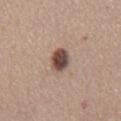Impression:
Recorded during total-body skin imaging; not selected for excision or biopsy.
Clinical summary:
The lesion is on the front of the torso. Captured under white-light illumination. The patient is a male in their mid- to late 50s. A lesion tile, about 15 mm wide, cut from a 3D total-body photograph.